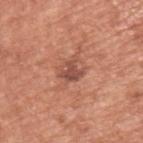{
  "biopsy_status": "not biopsied; imaged during a skin examination",
  "site": "left upper arm",
  "image": {
    "source": "total-body photography crop",
    "field_of_view_mm": 15
  },
  "patient": {
    "sex": "male",
    "age_approx": 65
  }
}